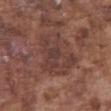workup: total-body-photography surveillance lesion; no biopsy
body site: the abdomen
diameter: ≈6 mm
acquisition: ~15 mm crop, total-body skin-cancer survey
subject: male, aged approximately 75